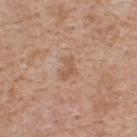The lesion was tiled from a total-body skin photograph and was not biopsied. A roughly 15 mm field-of-view crop from a total-body skin photograph. The tile uses white-light illumination. Approximately 2.5 mm at its widest. A male patient, about 60 years old. From the upper back.